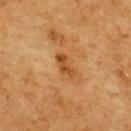  biopsy_status: not biopsied; imaged during a skin examination
  automated_metrics:
    shape_asymmetry: 0.35
    vs_skin_darker_L: 11.0
    vs_skin_contrast_norm: 8.0
    border_irregularity_0_10: 4.0
    color_variation_0_10: 2.0
    peripheral_color_asymmetry: 0.0
    lesion_detection_confidence_0_100: 100
  patient:
    sex: female
    age_approx: 55
  image:
    source: total-body photography crop
    field_of_view_mm: 15
  site: upper back
  lesion_size:
    long_diameter_mm_approx: 3.0